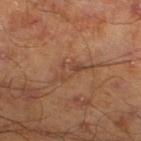workup — no biopsy performed (imaged during a skin exam); illumination — cross-polarized illumination; subject — male, aged around 45; site — the left lower leg; lesion size — ≈5 mm; image source — total-body-photography crop, ~15 mm field of view.